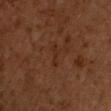Clinical impression: Recorded during total-body skin imaging; not selected for excision or biopsy. Acquisition and patient details: The recorded lesion diameter is about 3 mm. The lesion is on the chest. A male patient, aged approximately 50. Automated tile analysis of the lesion measured an automated nevus-likeness rating near 0 out of 100. Imaged with cross-polarized lighting. This image is a 15 mm lesion crop taken from a total-body photograph.